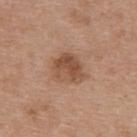The lesion was photographed on a routine skin check and not biopsied; there is no pathology result.
This is a white-light tile.
The lesion is located on the back.
A female subject aged 38–42.
The total-body-photography lesion software estimated an area of roughly 10 mm², an eccentricity of roughly 0.55, and a shape-asymmetry score of about 0.25 (0 = symmetric). The software also gave roughly 10 lightness units darker than nearby skin and a lesion-to-skin contrast of about 7.5 (normalized; higher = more distinct). And it measured a border-irregularity rating of about 2.5/10, internal color variation of about 5 on a 0–10 scale, and peripheral color asymmetry of about 2. And it measured an automated nevus-likeness rating near 45 out of 100 and lesion-presence confidence of about 100/100.
About 4 mm across.
A region of skin cropped from a whole-body photographic capture, roughly 15 mm wide.